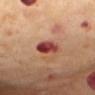Clinical impression:
The lesion was photographed on a routine skin check and not biopsied; there is no pathology result.
Background:
On the mid back. A 15 mm close-up tile from a total-body photography series done for melanoma screening. Measured at roughly 3 mm in maximum diameter. Imaged with cross-polarized lighting. A female subject, aged 53 to 57.